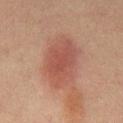notes: imaged on a skin check; not biopsied
imaging modality: total-body-photography crop, ~15 mm field of view
site: the mid back
patient: male, in their mid- to late 60s
illumination: cross-polarized
automated metrics: a lesion area of about 23 mm² and an eccentricity of roughly 0.8; a classifier nevus-likeness of about 95/100 and a lesion-detection confidence of about 100/100